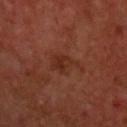Findings:
* biopsy status — no biopsy performed (imaged during a skin exam)